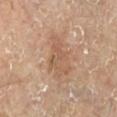Recorded during total-body skin imaging; not selected for excision or biopsy. A subject aged 53 to 57. From the left lower leg. About 5 mm across. The total-body-photography lesion software estimated a lesion area of about 11 mm² and a symmetry-axis asymmetry near 0.35. It also reported roughly 7 lightness units darker than nearby skin and a lesion-to-skin contrast of about 5.5 (normalized; higher = more distinct). A lesion tile, about 15 mm wide, cut from a 3D total-body photograph. Captured under cross-polarized illumination.